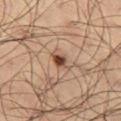Q: Was a biopsy performed?
A: no biopsy performed (imaged during a skin exam)
Q: What are the patient's age and sex?
A: male, aged 63 to 67
Q: Lesion location?
A: the left thigh
Q: What is the imaging modality?
A: ~15 mm tile from a whole-body skin photo